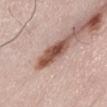{"biopsy_status": "not biopsied; imaged during a skin examination", "patient": {"sex": "female", "age_approx": 50}, "site": "abdomen", "image": {"source": "total-body photography crop", "field_of_view_mm": 15}, "lesion_size": {"long_diameter_mm_approx": 5.0}, "automated_metrics": {"area_mm2_approx": 7.5, "eccentricity": 0.9, "shape_asymmetry": 0.25, "vs_skin_darker_L": 17.0, "vs_skin_contrast_norm": 12.0, "color_variation_0_10": 5.5, "peripheral_color_asymmetry": 2.0}, "lighting": "white-light"}